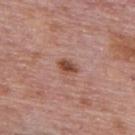Impression:
The lesion was photographed on a routine skin check and not biopsied; there is no pathology result.
Context:
A male patient aged approximately 75. This is a white-light tile. Cropped from a whole-body photographic skin survey; the tile spans about 15 mm. The lesion is on the upper back. The lesion's longest dimension is about 2.5 mm.